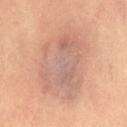Part of a total-body skin-imaging series; this lesion was reviewed on a skin check and was not flagged for biopsy. A female patient, aged 48 to 52. Imaged with cross-polarized lighting. A 15 mm close-up tile from a total-body photography series done for melanoma screening.